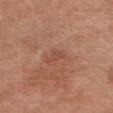Findings:
• workup · total-body-photography surveillance lesion; no biopsy
• acquisition · total-body-photography crop, ~15 mm field of view
• automated metrics · a border-irregularity rating of about 4.5/10, internal color variation of about 0.5 on a 0–10 scale, and peripheral color asymmetry of about 0
• tile lighting · white-light
• site · the chest
• subject · female, approximately 65 years of age